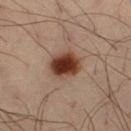This lesion was catalogued during total-body skin photography and was not selected for biopsy.